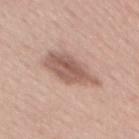The lesion was tiled from a total-body skin photograph and was not biopsied. The subject is a female aged approximately 55. A close-up tile cropped from a whole-body skin photograph, about 15 mm across. The lesion is located on the mid back.